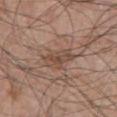Captured during whole-body skin photography for melanoma surveillance; the lesion was not biopsied. From the chest. Cropped from a whole-body photographic skin survey; the tile spans about 15 mm. The recorded lesion diameter is about 4.5 mm. The patient is a male aged 68–72.